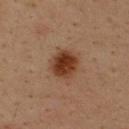On the upper back.
Approximately 4 mm at its widest.
A region of skin cropped from a whole-body photographic capture, roughly 15 mm wide.
The subject is a male in their mid-30s.
Imaged with cross-polarized lighting.